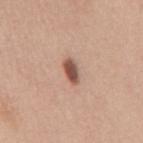| field | value |
|---|---|
| workup | total-body-photography surveillance lesion; no biopsy |
| subject | male, roughly 50 years of age |
| location | the mid back |
| automated lesion analysis | a lesion color around L≈53 a*≈21 b*≈27 in CIELAB, roughly 15 lightness units darker than nearby skin, and a normalized lesion–skin contrast near 10 |
| lighting | white-light illumination |
| lesion size | ≈3 mm |
| imaging modality | ~15 mm tile from a whole-body skin photo |